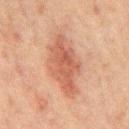workup: total-body-photography surveillance lesion; no biopsy | image source: total-body-photography crop, ~15 mm field of view | automated lesion analysis: about 9 CIELAB-L* units darker than the surrounding skin and a lesion-to-skin contrast of about 7 (normalized; higher = more distinct); a border-irregularity rating of about 3/10, a color-variation rating of about 4/10, and a peripheral color-asymmetry measure near 1.5 | site: the back | size: ~8 mm (longest diameter) | lighting: cross-polarized illumination | patient: male, approximately 65 years of age.